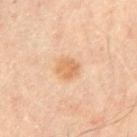Q: Was a biopsy performed?
A: catalogued during a skin exam; not biopsied
Q: What kind of image is this?
A: 15 mm crop, total-body photography
Q: What lighting was used for the tile?
A: cross-polarized illumination
Q: How large is the lesion?
A: ~3 mm (longest diameter)
Q: Who is the patient?
A: male, approximately 65 years of age
Q: What is the anatomic site?
A: the abdomen